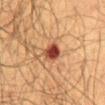Part of a total-body skin-imaging series; this lesion was reviewed on a skin check and was not flagged for biopsy.
A 15 mm crop from a total-body photograph taken for skin-cancer surveillance.
An algorithmic analysis of the crop reported an area of roughly 5.5 mm², a shape eccentricity near 0.4, and a symmetry-axis asymmetry near 0.2. It also reported a lesion color around L≈44 a*≈27 b*≈31 in CIELAB and a normalized lesion–skin contrast near 12. It also reported an automated nevus-likeness rating near 0 out of 100.
A male patient aged 58 to 62.
The tile uses cross-polarized illumination.
The lesion is on the abdomen.
About 2.5 mm across.